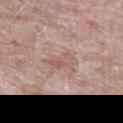Assessment:
No biopsy was performed on this lesion — it was imaged during a full skin examination and was not determined to be concerning.
Clinical summary:
This is a white-light tile. A male subject roughly 65 years of age. A 15 mm close-up tile from a total-body photography series done for melanoma screening. The lesion is on the mid back. The total-body-photography lesion software estimated a lesion area of about 4 mm² and a symmetry-axis asymmetry near 0.35. It also reported an average lesion color of about L≈56 a*≈20 b*≈25 (CIELAB) and a normalized lesion–skin contrast near 5.5. And it measured a border-irregularity rating of about 4/10, a within-lesion color-variation index near 2/10, and a peripheral color-asymmetry measure near 0. The analysis additionally found an automated nevus-likeness rating near 0 out of 100 and a lesion-detection confidence of about 100/100. Longest diameter approximately 3 mm.